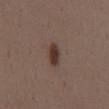This lesion was catalogued during total-body skin photography and was not selected for biopsy. Cropped from a whole-body photographic skin survey; the tile spans about 15 mm. Imaged with white-light lighting. The lesion is on the chest. A male patient aged approximately 40. Longest diameter approximately 3 mm.